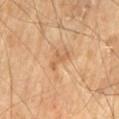Context: A lesion tile, about 15 mm wide, cut from a 3D total-body photograph. This is a cross-polarized tile. From the left thigh. The recorded lesion diameter is about 3 mm. A male patient in their 70s.